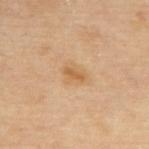The lesion was photographed on a routine skin check and not biopsied; there is no pathology result. Cropped from a total-body skin-imaging series; the visible field is about 15 mm. The lesion is on the upper back. The lesion's longest dimension is about 3 mm. The tile uses cross-polarized illumination. A female patient roughly 65 years of age.